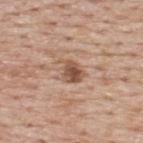<tbp_lesion>
  <biopsy_status>not biopsied; imaged during a skin examination</biopsy_status>
  <patient>
    <sex>male</sex>
    <age_approx>75</age_approx>
  </patient>
  <image>
    <source>total-body photography crop</source>
    <field_of_view_mm>15</field_of_view_mm>
  </image>
  <site>upper back</site>
</tbp_lesion>